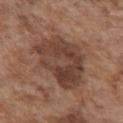Context: The lesion is on the front of the torso. A male subject, aged approximately 75. A 15 mm close-up tile from a total-body photography series done for melanoma screening. The lesion's longest dimension is about 7.5 mm.